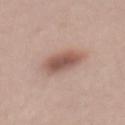Impression: Captured during whole-body skin photography for melanoma surveillance; the lesion was not biopsied. Acquisition and patient details: A female subject, aged 38 to 42. The tile uses white-light illumination. Cropped from a whole-body photographic skin survey; the tile spans about 15 mm. Approximately 5 mm at its widest. On the mid back.